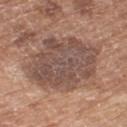Recorded during total-body skin imaging; not selected for excision or biopsy. Measured at roughly 7.5 mm in maximum diameter. This image is a 15 mm lesion crop taken from a total-body photograph. A female subject roughly 75 years of age. From the left thigh.